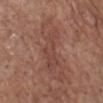This lesion was catalogued during total-body skin photography and was not selected for biopsy. Automated tile analysis of the lesion measured an area of roughly 10 mm², a shape eccentricity near 0.95, and a shape-asymmetry score of about 0.45 (0 = symmetric). The analysis additionally found a border-irregularity index near 6/10 and internal color variation of about 2.5 on a 0–10 scale. The analysis additionally found an automated nevus-likeness rating near 0 out of 100 and a detector confidence of about 80 out of 100 that the crop contains a lesion. The recorded lesion diameter is about 6.5 mm. A male subject, aged 68 to 72. A 15 mm crop from a total-body photograph taken for skin-cancer surveillance. The lesion is on the chest. Imaged with white-light lighting.